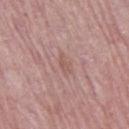Part of a total-body skin-imaging series; this lesion was reviewed on a skin check and was not flagged for biopsy.
From the right thigh.
Imaged with white-light lighting.
An algorithmic analysis of the crop reported a lesion color around L≈55 a*≈20 b*≈24 in CIELAB, a lesion–skin lightness drop of about 6, and a normalized lesion–skin contrast near 5. The analysis additionally found a border-irregularity rating of about 3.5/10, internal color variation of about 0 on a 0–10 scale, and radial color variation of about 0. And it measured an automated nevus-likeness rating near 0 out of 100 and lesion-presence confidence of about 95/100.
The subject is a female about 65 years old.
The lesion's longest dimension is about 2.5 mm.
A 15 mm close-up extracted from a 3D total-body photography capture.